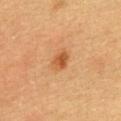Clinical impression:
Recorded during total-body skin imaging; not selected for excision or biopsy.
Background:
A 15 mm close-up extracted from a 3D total-body photography capture. This is a cross-polarized tile. From the back. A female patient, about 30 years old.